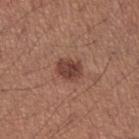Recorded during total-body skin imaging; not selected for excision or biopsy.
The subject is a male aged around 40.
Longest diameter approximately 3 mm.
Imaged with white-light lighting.
A 15 mm close-up tile from a total-body photography series done for melanoma screening.
From the left forearm.